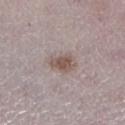Imaged during a routine full-body skin examination; the lesion was not biopsied and no histopathology is available. The lesion is on the left lower leg. A 15 mm close-up extracted from a 3D total-body photography capture. The total-body-photography lesion software estimated an average lesion color of about L≈54 a*≈14 b*≈21 (CIELAB), a lesion–skin lightness drop of about 10, and a normalized border contrast of about 7.5. It also reported a within-lesion color-variation index near 3.5/10 and radial color variation of about 1. A female subject, aged 48–52.